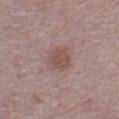follow-up: no biopsy performed (imaged during a skin exam)
location: the abdomen
subject: male, approximately 75 years of age
acquisition: ~15 mm tile from a whole-body skin photo
lesion size: ~3.5 mm (longest diameter)